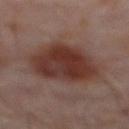Q: Was a biopsy performed?
A: imaged on a skin check; not biopsied
Q: How large is the lesion?
A: ≈6.5 mm
Q: Lesion location?
A: the front of the torso
Q: Automated lesion metrics?
A: an area of roughly 29 mm², an eccentricity of roughly 0.45, and a shape-asymmetry score of about 0.2 (0 = symmetric)
Q: Who is the patient?
A: male, about 60 years old
Q: How was the tile lit?
A: cross-polarized illumination
Q: What is the imaging modality?
A: ~15 mm crop, total-body skin-cancer survey